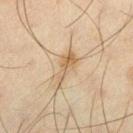Recorded during total-body skin imaging; not selected for excision or biopsy.
From the left thigh.
A male patient, about 45 years old.
A lesion tile, about 15 mm wide, cut from a 3D total-body photograph.